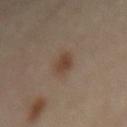Assessment: Part of a total-body skin-imaging series; this lesion was reviewed on a skin check and was not flagged for biopsy. Background: The lesion is located on the mid back. A female patient aged approximately 60. Cropped from a whole-body photographic skin survey; the tile spans about 15 mm. Measured at roughly 3.5 mm in maximum diameter.